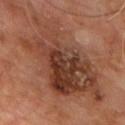Q: Was this lesion biopsied?
A: total-body-photography surveillance lesion; no biopsy
Q: Where on the body is the lesion?
A: the upper back
Q: Illumination type?
A: cross-polarized
Q: What kind of image is this?
A: ~15 mm crop, total-body skin-cancer survey
Q: How large is the lesion?
A: ~12.5 mm (longest diameter)
Q: What are the patient's age and sex?
A: male, aged approximately 60